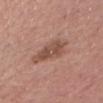Notes:
* biopsy status: total-body-photography surveillance lesion; no biopsy
* patient: male, aged 68–72
* lesion diameter: ~4.5 mm (longest diameter)
* automated lesion analysis: an area of roughly 11 mm², an outline eccentricity of about 0.75 (0 = round, 1 = elongated), and two-axis asymmetry of about 0.35; a mean CIELAB color near L≈51 a*≈21 b*≈27 and a normalized border contrast of about 7; a nevus-likeness score of about 10/100 and a detector confidence of about 100 out of 100 that the crop contains a lesion
* illumination: white-light
* imaging modality: total-body-photography crop, ~15 mm field of view
* location: the chest